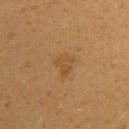A 15 mm close-up extracted from a 3D total-body photography capture. The lesion's longest dimension is about 3 mm. A female subject about 20 years old. From the upper back. Automated image analysis of the tile measured a footprint of about 5 mm² and a symmetry-axis asymmetry near 0.35. The analysis additionally found a mean CIELAB color near L≈43 a*≈16 b*≈34, about 5 CIELAB-L* units darker than the surrounding skin, and a normalized lesion–skin contrast near 5. And it measured a border-irregularity rating of about 3.5/10, a within-lesion color-variation index near 2/10, and a peripheral color-asymmetry measure near 0.5. And it measured a classifier nevus-likeness of about 10/100 and lesion-presence confidence of about 100/100.